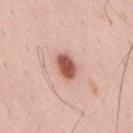Imaged during a routine full-body skin examination; the lesion was not biopsied and no histopathology is available. This is a white-light tile. An algorithmic analysis of the crop reported a lesion color around L≈56 a*≈26 b*≈28 in CIELAB, about 18 CIELAB-L* units darker than the surrounding skin, and a normalized lesion–skin contrast near 11. About 3.5 mm across. Located on the back. A male subject, aged around 35. A region of skin cropped from a whole-body photographic capture, roughly 15 mm wide.